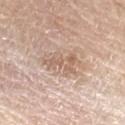workup: catalogued during a skin exam; not biopsied | illumination: white-light illumination | anatomic site: the left forearm | automated lesion analysis: about 9 CIELAB-L* units darker than the surrounding skin and a lesion-to-skin contrast of about 6 (normalized; higher = more distinct) | subject: male, approximately 80 years of age | imaging modality: ~15 mm crop, total-body skin-cancer survey.